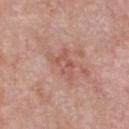biopsy status: imaged on a skin check; not biopsied
acquisition: 15 mm crop, total-body photography
patient: male, in their mid- to late 50s
site: the chest